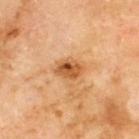Q: Is there a histopathology result?
A: catalogued during a skin exam; not biopsied
Q: What did automated image analysis measure?
A: a footprint of about 6 mm², an eccentricity of roughly 0.7, and a shape-asymmetry score of about 0.2 (0 = symmetric)
Q: What is the anatomic site?
A: the upper back
Q: What kind of image is this?
A: ~15 mm crop, total-body skin-cancer survey
Q: How was the tile lit?
A: cross-polarized illumination
Q: Who is the patient?
A: male, aged 68 to 72